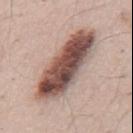* patient: male, aged approximately 55
* image source: 15 mm crop, total-body photography
* site: the mid back